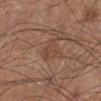Q: Is there a histopathology result?
A: total-body-photography surveillance lesion; no biopsy
Q: What are the patient's age and sex?
A: male, aged around 55
Q: How was this image acquired?
A: 15 mm crop, total-body photography
Q: How large is the lesion?
A: ~3 mm (longest diameter)
Q: Where on the body is the lesion?
A: the left lower leg
Q: Automated lesion metrics?
A: a lesion color around L≈45 a*≈20 b*≈27 in CIELAB and a normalized border contrast of about 4.5; a border-irregularity index near 5/10 and peripheral color asymmetry of about 0.5; a classifier nevus-likeness of about 0/100 and lesion-presence confidence of about 100/100